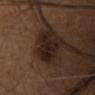Case summary:
• biopsy status: total-body-photography surveillance lesion; no biopsy
• body site: the upper back
• acquisition: ~15 mm crop, total-body skin-cancer survey
• TBP lesion metrics: a lesion area of about 10 mm², an eccentricity of roughly 0.45, and a symmetry-axis asymmetry near 0.3; a border-irregularity index near 3.5/10, a color-variation rating of about 3.5/10, and radial color variation of about 1; an automated nevus-likeness rating near 10 out of 100 and a lesion-detection confidence of about 100/100
• subject: female, approximately 40 years of age
• lesion size: ~4 mm (longest diameter)